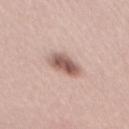Impression: No biopsy was performed on this lesion — it was imaged during a full skin examination and was not determined to be concerning. Acquisition and patient details: The lesion-visualizer software estimated an eccentricity of roughly 0.8. The software also gave a lesion color around L≈57 a*≈20 b*≈23 in CIELAB, about 16 CIELAB-L* units darker than the surrounding skin, and a lesion-to-skin contrast of about 10 (normalized; higher = more distinct). The analysis additionally found a border-irregularity rating of about 2/10, a color-variation rating of about 5/10, and a peripheral color-asymmetry measure near 1.5. It also reported a classifier nevus-likeness of about 95/100 and a detector confidence of about 100 out of 100 that the crop contains a lesion. A 15 mm close-up tile from a total-body photography series done for melanoma screening. The subject is a male aged 28–32. The lesion's longest dimension is about 4 mm.